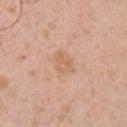biopsy_status: not biopsied; imaged during a skin examination
site: chest
lesion_size:
  long_diameter_mm_approx: 3.0
lighting: white-light
automated_metrics:
  area_mm2_approx: 4.5
  eccentricity: 0.7
  shape_asymmetry: 0.3
  border_irregularity_0_10: 3.0
  color_variation_0_10: 2.0
  peripheral_color_asymmetry: 1.0
  nevus_likeness_0_100: 0
  lesion_detection_confidence_0_100: 100
patient:
  sex: male
  age_approx: 30
image:
  source: total-body photography crop
  field_of_view_mm: 15